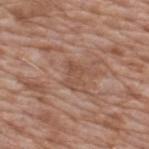illumination=white-light
imaging modality=~15 mm crop, total-body skin-cancer survey
TBP lesion metrics=a lesion color around L≈50 a*≈20 b*≈28 in CIELAB, roughly 6 lightness units darker than nearby skin, and a normalized border contrast of about 5; a nevus-likeness score of about 0/100 and lesion-presence confidence of about 100/100
patient=male, approximately 60 years of age
anatomic site=the back